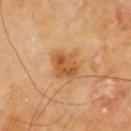notes=catalogued during a skin exam; not biopsied | site=the right upper arm | size=about 3.5 mm | image-analysis metrics=a lesion color around L≈56 a*≈24 b*≈42 in CIELAB and about 11 CIELAB-L* units darker than the surrounding skin; a peripheral color-asymmetry measure near 1.5; a classifier nevus-likeness of about 65/100 | subject=male, in their mid- to late 60s | image source=~15 mm tile from a whole-body skin photo.